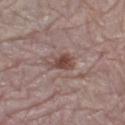Impression: Imaged during a routine full-body skin examination; the lesion was not biopsied and no histopathology is available. Image and clinical context: A 15 mm close-up extracted from a 3D total-body photography capture. A female patient, roughly 70 years of age. The lesion's longest dimension is about 3.5 mm. On the left lower leg. The lesion-visualizer software estimated an area of roughly 5 mm², a shape eccentricity near 0.85, and a shape-asymmetry score of about 0.4 (0 = symmetric). It also reported a mean CIELAB color near L≈46 a*≈19 b*≈22 and a lesion–skin lightness drop of about 11. And it measured internal color variation of about 3 on a 0–10 scale.